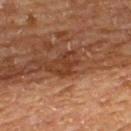follow-up: imaged on a skin check; not biopsied | lighting: cross-polarized | patient: male, aged approximately 65 | site: the upper back | diameter: ~3 mm (longest diameter) | acquisition: ~15 mm crop, total-body skin-cancer survey.